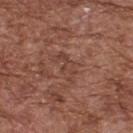No biopsy was performed on this lesion — it was imaged during a full skin examination and was not determined to be concerning.
A region of skin cropped from a whole-body photographic capture, roughly 15 mm wide.
The recorded lesion diameter is about 3.5 mm.
The subject is a male aged approximately 75.
The tile uses white-light illumination.
The lesion is located on the upper back.